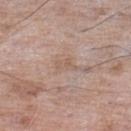Notes:
* subject: male, aged 73 to 77
* image: total-body-photography crop, ~15 mm field of view
* body site: the left lower leg